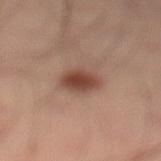No biopsy was performed on this lesion — it was imaged during a full skin examination and was not determined to be concerning.
On the left lower leg.
About 3.5 mm across.
Automated tile analysis of the lesion measured a mean CIELAB color near L≈43 a*≈21 b*≈26, about 12 CIELAB-L* units darker than the surrounding skin, and a lesion-to-skin contrast of about 9.5 (normalized; higher = more distinct). And it measured a classifier nevus-likeness of about 100/100 and a detector confidence of about 100 out of 100 that the crop contains a lesion.
A male subject aged around 40.
Cropped from a total-body skin-imaging series; the visible field is about 15 mm.
Imaged with cross-polarized lighting.